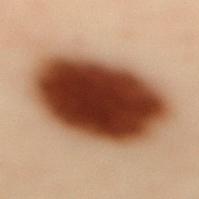biopsy status — total-body-photography surveillance lesion; no biopsy
patient — female, approximately 60 years of age
location — the mid back
image — ~15 mm crop, total-body skin-cancer survey